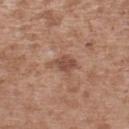Assessment: Part of a total-body skin-imaging series; this lesion was reviewed on a skin check and was not flagged for biopsy. Clinical summary: A region of skin cropped from a whole-body photographic capture, roughly 15 mm wide. The total-body-photography lesion software estimated a lesion color around L≈49 a*≈21 b*≈28 in CIELAB, roughly 10 lightness units darker than nearby skin, and a lesion-to-skin contrast of about 7 (normalized; higher = more distinct). And it measured border irregularity of about 3.5 on a 0–10 scale. It also reported lesion-presence confidence of about 100/100. The subject is a male about 45 years old. The lesion is located on the left upper arm.